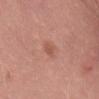Assessment: Captured during whole-body skin photography for melanoma surveillance; the lesion was not biopsied. Image and clinical context: A 15 mm close-up extracted from a 3D total-body photography capture. The lesion is on the lower back. The subject is a male about 40 years old.